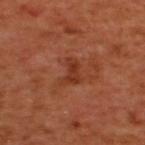Recorded during total-body skin imaging; not selected for excision or biopsy.
Imaged with cross-polarized lighting.
An algorithmic analysis of the crop reported a mean CIELAB color near L≈36 a*≈28 b*≈34 and a lesion–skin lightness drop of about 8. The analysis additionally found border irregularity of about 5.5 on a 0–10 scale, internal color variation of about 2.5 on a 0–10 scale, and radial color variation of about 1.
Located on the upper back.
Cropped from a total-body skin-imaging series; the visible field is about 15 mm.
The lesion's longest dimension is about 3 mm.
The subject is a male roughly 50 years of age.